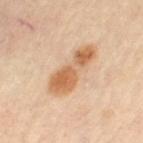Clinical impression: No biopsy was performed on this lesion — it was imaged during a full skin examination and was not determined to be concerning. Image and clinical context: A 15 mm close-up extracted from a 3D total-body photography capture. The lesion is on the leg. The patient is a female aged 63–67.